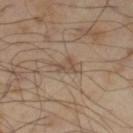notes = catalogued during a skin exam; not biopsied
subject = male, aged approximately 55
imaging modality = total-body-photography crop, ~15 mm field of view
diameter = about 2.5 mm
lighting = cross-polarized
location = the left thigh